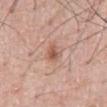Part of a total-body skin-imaging series; this lesion was reviewed on a skin check and was not flagged for biopsy.